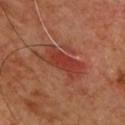Findings:
• body site: the chest
• automated metrics: a mean CIELAB color near L≈40 a*≈30 b*≈31, a lesion–skin lightness drop of about 9, and a lesion-to-skin contrast of about 7 (normalized; higher = more distinct); border irregularity of about 5 on a 0–10 scale
• imaging modality: 15 mm crop, total-body photography
• subject: male, aged 48 to 52
• tile lighting: cross-polarized